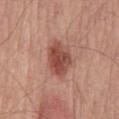Captured during whole-body skin photography for melanoma surveillance; the lesion was not biopsied.
Measured at roughly 4 mm in maximum diameter.
A male subject aged 68–72.
A 15 mm crop from a total-body photograph taken for skin-cancer surveillance.
The lesion is located on the mid back.
Captured under white-light illumination.